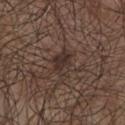Clinical impression: This lesion was catalogued during total-body skin photography and was not selected for biopsy. Image and clinical context: Longest diameter approximately 3 mm. Imaged with white-light lighting. A male patient in their mid- to late 50s. A close-up tile cropped from a whole-body skin photograph, about 15 mm across. From the front of the torso.